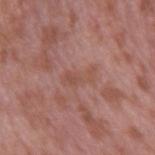Imaged during a routine full-body skin examination; the lesion was not biopsied and no histopathology is available.
Cropped from a total-body skin-imaging series; the visible field is about 15 mm.
A male patient, aged around 40.
Captured under white-light illumination.
The lesion is located on the right upper arm.